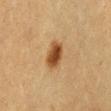Assessment: Part of a total-body skin-imaging series; this lesion was reviewed on a skin check and was not flagged for biopsy.